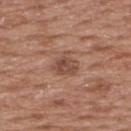Clinical impression: No biopsy was performed on this lesion — it was imaged during a full skin examination and was not determined to be concerning. Context: From the upper back. Measured at roughly 3 mm in maximum diameter. The lesion-visualizer software estimated a lesion area of about 7 mm² and a shape eccentricity near 0.4. It also reported a lesion color around L≈48 a*≈21 b*≈27 in CIELAB, roughly 9 lightness units darker than nearby skin, and a lesion-to-skin contrast of about 7 (normalized; higher = more distinct). The analysis additionally found an automated nevus-likeness rating near 15 out of 100. This image is a 15 mm lesion crop taken from a total-body photograph. The subject is a male approximately 55 years of age. This is a white-light tile.